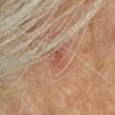Notes:
* follow-up — no biopsy performed (imaged during a skin exam)
* patient — female, roughly 75 years of age
* image source — total-body-photography crop, ~15 mm field of view
* body site — the head or neck
* diameter — about 2.5 mm